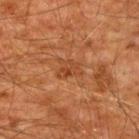Captured during whole-body skin photography for melanoma surveillance; the lesion was not biopsied. The lesion is on the upper back. Automated tile analysis of the lesion measured an area of roughly 5 mm² and an outline eccentricity of about 0.65 (0 = round, 1 = elongated). The analysis additionally found border irregularity of about 4 on a 0–10 scale, a color-variation rating of about 3/10, and peripheral color asymmetry of about 1. The software also gave an automated nevus-likeness rating near 0 out of 100. About 3 mm across. A roughly 15 mm field-of-view crop from a total-body skin photograph. A male patient, approximately 60 years of age. This is a cross-polarized tile.